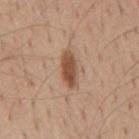follow-up = no biopsy performed (imaged during a skin exam) | size = ~4.5 mm (longest diameter) | subject = male, in their mid- to late 50s | site = the mid back | image = ~15 mm tile from a whole-body skin photo | lighting = white-light | automated metrics = a lesion color around L≈50 a*≈21 b*≈31 in CIELAB and a lesion–skin lightness drop of about 14; a border-irregularity index near 3/10, a color-variation rating of about 3/10, and peripheral color asymmetry of about 1; lesion-presence confidence of about 100/100.